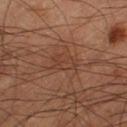No biopsy was performed on this lesion — it was imaged during a full skin examination and was not determined to be concerning. Located on the left thigh. A 15 mm close-up extracted from a 3D total-body photography capture. The tile uses cross-polarized illumination. The subject is a male in their 60s. Approximately 3 mm at its widest.